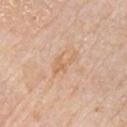{
  "biopsy_status": "not biopsied; imaged during a skin examination",
  "lighting": "white-light",
  "site": "chest",
  "automated_metrics": {
    "area_mm2_approx": 4.0,
    "eccentricity": 0.9,
    "shape_asymmetry": 0.55,
    "border_irregularity_0_10": 7.5,
    "color_variation_0_10": 1.0,
    "peripheral_color_asymmetry": 0.5,
    "nevus_likeness_0_100": 0
  },
  "patient": {
    "sex": "male",
    "age_approx": 75
  },
  "lesion_size": {
    "long_diameter_mm_approx": 4.0
  },
  "image": {
    "source": "total-body photography crop",
    "field_of_view_mm": 15
  }
}